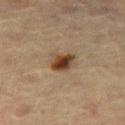<tbp_lesion>
  <biopsy_status>not biopsied; imaged during a skin examination</biopsy_status>
  <patient>
    <sex>female</sex>
    <age_approx>65</age_approx>
  </patient>
  <site>right thigh</site>
  <lighting>cross-polarized</lighting>
  <automated_metrics>
    <area_mm2_approx>6.0</area_mm2_approx>
    <eccentricity>0.65</eccentricity>
    <shape_asymmetry>0.3</shape_asymmetry>
    <nevus_likeness_0_100>100</nevus_likeness_0_100>
    <lesion_detection_confidence_0_100>100</lesion_detection_confidence_0_100>
  </automated_metrics>
  <image>
    <source>total-body photography crop</source>
    <field_of_view_mm>15</field_of_view_mm>
  </image>
  <lesion_size>
    <long_diameter_mm_approx>3.0</long_diameter_mm_approx>
  </lesion_size>
</tbp_lesion>